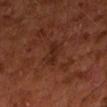workup = no biopsy performed (imaged during a skin exam); patient = male, about 60 years old; site = the left forearm; size = ≈3.5 mm; tile lighting = cross-polarized illumination; imaging modality = ~15 mm tile from a whole-body skin photo.